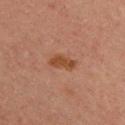Assessment:
Part of a total-body skin-imaging series; this lesion was reviewed on a skin check and was not flagged for biopsy.
Background:
From the back. Cropped from a whole-body photographic skin survey; the tile spans about 15 mm. This is a cross-polarized tile. Measured at roughly 3 mm in maximum diameter. A male subject aged around 30.